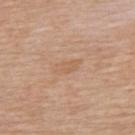<lesion>
  <biopsy_status>not biopsied; imaged during a skin examination</biopsy_status>
  <image>
    <source>total-body photography crop</source>
    <field_of_view_mm>15</field_of_view_mm>
  </image>
  <lighting>white-light</lighting>
  <automated_metrics>
    <nevus_likeness_0_100>0</nevus_likeness_0_100>
    <lesion_detection_confidence_0_100>100</lesion_detection_confidence_0_100>
  </automated_metrics>
  <lesion_size>
    <long_diameter_mm_approx>3.5</long_diameter_mm_approx>
  </lesion_size>
  <patient>
    <sex>male</sex>
    <age_approx>65</age_approx>
  </patient>
  <site>upper back</site>
</lesion>